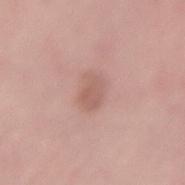Recorded during total-body skin imaging; not selected for excision or biopsy.
A 15 mm close-up extracted from a 3D total-body photography capture.
A male patient, aged 38–42.
The lesion is on the lower back.